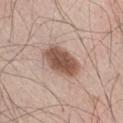Q: Was this lesion biopsied?
A: imaged on a skin check; not biopsied
Q: What are the patient's age and sex?
A: male, in their mid-40s
Q: How was this image acquired?
A: ~15 mm tile from a whole-body skin photo
Q: How was the tile lit?
A: white-light illumination
Q: Where on the body is the lesion?
A: the right thigh
Q: What is the lesion's diameter?
A: ≈5 mm